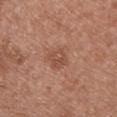Captured during whole-body skin photography for melanoma surveillance; the lesion was not biopsied. The total-body-photography lesion software estimated a footprint of about 5 mm² and an outline eccentricity of about 0.5 (0 = round, 1 = elongated). The analysis additionally found an average lesion color of about L≈50 a*≈23 b*≈29 (CIELAB), roughly 8 lightness units darker than nearby skin, and a lesion-to-skin contrast of about 5.5 (normalized; higher = more distinct). It also reported a border-irregularity index near 2/10 and a within-lesion color-variation index near 3.5/10. It also reported a classifier nevus-likeness of about 5/100 and a lesion-detection confidence of about 100/100. The tile uses white-light illumination. A region of skin cropped from a whole-body photographic capture, roughly 15 mm wide. The lesion is located on the chest. About 2.5 mm across. The patient is a female aged 38–42.